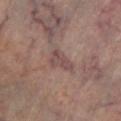Assessment: This lesion was catalogued during total-body skin photography and was not selected for biopsy. Context: Approximately 3.5 mm at its widest. This is a cross-polarized tile. Cropped from a total-body skin-imaging series; the visible field is about 15 mm. On the left lower leg.